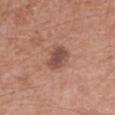No biopsy was performed on this lesion — it was imaged during a full skin examination and was not determined to be concerning.
Approximately 3.5 mm at its widest.
From the left forearm.
A 15 mm close-up extracted from a 3D total-body photography capture.
A female patient, aged 38–42.
Captured under white-light illumination.